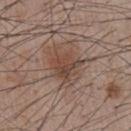follow-up=catalogued during a skin exam; not biopsied | subject=male, roughly 55 years of age | diameter=≈3.5 mm | image-analysis metrics=a lesion color around L≈44 a*≈18 b*≈25 in CIELAB, a lesion–skin lightness drop of about 9, and a normalized border contrast of about 7 | image source=~15 mm crop, total-body skin-cancer survey | body site=the chest | lighting=white-light illumination.